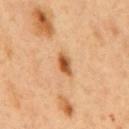Impression: Recorded during total-body skin imaging; not selected for excision or biopsy. Acquisition and patient details: A 15 mm close-up extracted from a 3D total-body photography capture. A male patient, roughly 50 years of age. From the back. Longest diameter approximately 3 mm. Imaged with cross-polarized lighting.